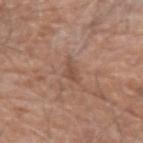Clinical impression:
Recorded during total-body skin imaging; not selected for excision or biopsy.
Image and clinical context:
Cropped from a whole-body photographic skin survey; the tile spans about 15 mm. Located on the arm. A male subject aged 78 to 82. Automated tile analysis of the lesion measured a footprint of about 3 mm², an eccentricity of roughly 0.9, and a shape-asymmetry score of about 0.5 (0 = symmetric). The analysis additionally found a border-irregularity rating of about 5.5/10 and a within-lesion color-variation index near 0.5/10. The analysis additionally found an automated nevus-likeness rating near 0 out of 100 and lesion-presence confidence of about 90/100. About 3.5 mm across. Captured under white-light illumination.